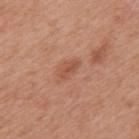Q: Was this lesion biopsied?
A: no biopsy performed (imaged during a skin exam)
Q: What lighting was used for the tile?
A: white-light illumination
Q: What is the lesion's diameter?
A: ~2.5 mm (longest diameter)
Q: What kind of image is this?
A: 15 mm crop, total-body photography
Q: Where on the body is the lesion?
A: the back
Q: Who is the patient?
A: male, aged around 70